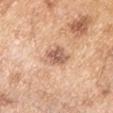Captured under white-light illumination. Approximately 3.5 mm at its widest. The subject is a male about 55 years old. A 15 mm crop from a total-body photograph taken for skin-cancer surveillance. From the arm.